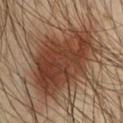Imaged during a routine full-body skin examination; the lesion was not biopsied and no histopathology is available.
From the chest.
Longest diameter approximately 9.5 mm.
A close-up tile cropped from a whole-body skin photograph, about 15 mm across.
Imaged with cross-polarized lighting.
The lesion-visualizer software estimated a lesion color around L≈39 a*≈22 b*≈29 in CIELAB, a lesion–skin lightness drop of about 12, and a normalized border contrast of about 10. And it measured border irregularity of about 3.5 on a 0–10 scale, internal color variation of about 5 on a 0–10 scale, and peripheral color asymmetry of about 1.5. The analysis additionally found an automated nevus-likeness rating near 95 out of 100 and a detector confidence of about 100 out of 100 that the crop contains a lesion.
A male patient about 65 years old.